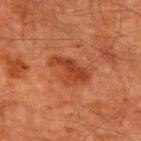follow-up=catalogued during a skin exam; not biopsied | subject=male, approximately 60 years of age | lesion diameter=~4.5 mm (longest diameter) | tile lighting=cross-polarized | imaging modality=~15 mm crop, total-body skin-cancer survey | location=the upper back.